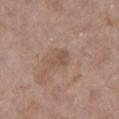Assessment:
This lesion was catalogued during total-body skin photography and was not selected for biopsy.
Image and clinical context:
This is a white-light tile. A female patient aged approximately 85 or older. From the front of the torso. Approximately 3 mm at its widest. A close-up tile cropped from a whole-body skin photograph, about 15 mm across.